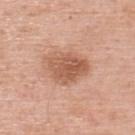Captured during whole-body skin photography for melanoma surveillance; the lesion was not biopsied. Cropped from a whole-body photographic skin survey; the tile spans about 15 mm. The subject is a male aged 58–62. The lesion-visualizer software estimated a lesion color around L≈57 a*≈23 b*≈32 in CIELAB, roughly 11 lightness units darker than nearby skin, and a normalized border contrast of about 7.5. The analysis additionally found border irregularity of about 2.5 on a 0–10 scale, internal color variation of about 4 on a 0–10 scale, and radial color variation of about 1.5. On the back. The tile uses white-light illumination. The recorded lesion diameter is about 5 mm.